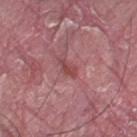follow-up = total-body-photography surveillance lesion; no biopsy
size = about 2.5 mm
subject = male, aged 38–42
site = the left thigh
imaging modality = ~15 mm tile from a whole-body skin photo